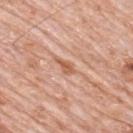{
  "biopsy_status": "not biopsied; imaged during a skin examination",
  "site": "upper back",
  "lesion_size": {
    "long_diameter_mm_approx": 2.5
  },
  "patient": {
    "sex": "male",
    "age_approx": 80
  },
  "automated_metrics": {
    "eccentricity": 0.85,
    "shape_asymmetry": 0.45,
    "border_irregularity_0_10": 4.0,
    "color_variation_0_10": 1.0
  },
  "image": {
    "source": "total-body photography crop",
    "field_of_view_mm": 15
  }
}